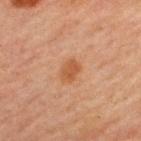{"biopsy_status": "not biopsied; imaged during a skin examination", "image": {"source": "total-body photography crop", "field_of_view_mm": 15}, "site": "upper back", "patient": {"sex": "male", "age_approx": 60}, "lighting": "cross-polarized", "lesion_size": {"long_diameter_mm_approx": 2.5}}